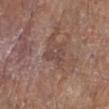Q: Is there a histopathology result?
A: no biopsy performed (imaged during a skin exam)
Q: What did automated image analysis measure?
A: a lesion color around L≈45 a*≈18 b*≈23 in CIELAB and roughly 6 lightness units darker than nearby skin; border irregularity of about 8 on a 0–10 scale, a color-variation rating of about 0/10, and a peripheral color-asymmetry measure near 0; an automated nevus-likeness rating near 0 out of 100 and lesion-presence confidence of about 95/100
Q: How was the tile lit?
A: white-light illumination
Q: What is the anatomic site?
A: the right lower leg
Q: Lesion size?
A: ~3 mm (longest diameter)
Q: What is the imaging modality?
A: total-body-photography crop, ~15 mm field of view
Q: Patient demographics?
A: female, in their 80s